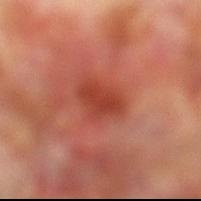The lesion is located on the left lower leg. A 15 mm crop from a total-body photograph taken for skin-cancer surveillance. Imaged with cross-polarized lighting. The lesion-visualizer software estimated an area of roughly 8.5 mm², an eccentricity of roughly 0.75, and a shape-asymmetry score of about 0.35 (0 = symmetric). The analysis additionally found border irregularity of about 3 on a 0–10 scale and a color-variation rating of about 2/10. It also reported an automated nevus-likeness rating near 35 out of 100. A male subject, approximately 70 years of age.